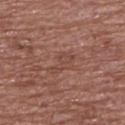Clinical impression:
The lesion was tiled from a total-body skin photograph and was not biopsied.
Context:
A 15 mm close-up extracted from a 3D total-body photography capture. The recorded lesion diameter is about 3.5 mm. The lesion is located on the upper back. A male patient, about 55 years old. This is a white-light tile.